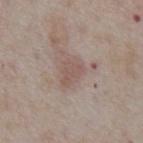Assessment:
Imaged during a routine full-body skin examination; the lesion was not biopsied and no histopathology is available.
Clinical summary:
On the abdomen. A close-up tile cropped from a whole-body skin photograph, about 15 mm across. A male patient, in their mid- to late 70s. The lesion's longest dimension is about 3 mm. This is a white-light tile.